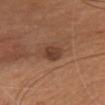{
  "biopsy_status": "not biopsied; imaged during a skin examination",
  "image": {
    "source": "total-body photography crop",
    "field_of_view_mm": 15
  },
  "lesion_size": {
    "long_diameter_mm_approx": 2.5
  },
  "automated_metrics": {
    "peripheral_color_asymmetry": 1.0
  },
  "lighting": "white-light",
  "patient": {
    "sex": "female",
    "age_approx": 45
  },
  "site": "chest"
}